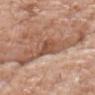workup: catalogued during a skin exam; not biopsied
patient: male, aged 73–77
site: the head or neck
acquisition: 15 mm crop, total-body photography